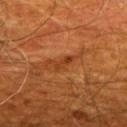This lesion was catalogued during total-body skin photography and was not selected for biopsy. On the upper back. A 15 mm crop from a total-body photograph taken for skin-cancer surveillance. A male subject approximately 60 years of age.